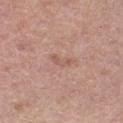This lesion was catalogued during total-body skin photography and was not selected for biopsy. The tile uses white-light illumination. The recorded lesion diameter is about 2.5 mm. The patient is a female in their 40s. This image is a 15 mm lesion crop taken from a total-body photograph. Located on the right thigh.